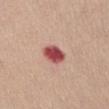  biopsy_status: not biopsied; imaged during a skin examination
  image:
    source: total-body photography crop
    field_of_view_mm: 15
  lighting: white-light
  site: abdomen
  lesion_size:
    long_diameter_mm_approx: 3.0
  automated_metrics:
    area_mm2_approx: 6.5
    eccentricity: 0.6
    shape_asymmetry: 0.15
  patient:
    sex: female
    age_approx: 75